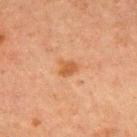The lesion-visualizer software estimated an area of roughly 4 mm², a shape eccentricity near 0.65, and two-axis asymmetry of about 0.35. It also reported a border-irregularity rating of about 3/10 and peripheral color asymmetry of about 0.5.
This image is a 15 mm lesion crop taken from a total-body photograph.
From the front of the torso.
The patient is a male aged 63 to 67.
Captured under cross-polarized illumination.
About 2.5 mm across.